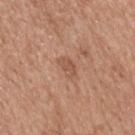Q: Is there a histopathology result?
A: total-body-photography surveillance lesion; no biopsy
Q: What is the imaging modality?
A: 15 mm crop, total-body photography
Q: Patient demographics?
A: male, roughly 65 years of age
Q: Lesion location?
A: the head or neck